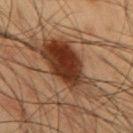workup = imaged on a skin check; not biopsied | automated metrics = an area of roughly 30 mm² and a symmetry-axis asymmetry near 0.4; a lesion color around L≈33 a*≈18 b*≈27 in CIELAB, roughly 13 lightness units darker than nearby skin, and a normalized lesion–skin contrast near 11.5; a border-irregularity index near 6.5/10 and a within-lesion color-variation index near 8/10; a lesion-detection confidence of about 100/100 | lesion diameter = ~10.5 mm (longest diameter) | acquisition = 15 mm crop, total-body photography | patient = male, about 55 years old | illumination = cross-polarized | site = the arm.